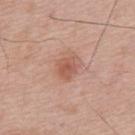A region of skin cropped from a whole-body photographic capture, roughly 15 mm wide.
Located on the back.
The patient is a male roughly 65 years of age.